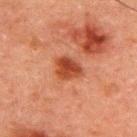follow-up=total-body-photography surveillance lesion; no biopsy
diameter=~3 mm (longest diameter)
image-analysis metrics=an area of roughly 7 mm²; a normalized lesion–skin contrast near 9; a border-irregularity rating of about 2/10
location=the upper back
subject=male, in their 50s
imaging modality=total-body-photography crop, ~15 mm field of view
illumination=cross-polarized illumination